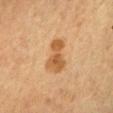No biopsy was performed on this lesion — it was imaged during a full skin examination and was not determined to be concerning. The lesion is located on the chest. The recorded lesion diameter is about 4.5 mm. A 15 mm close-up tile from a total-body photography series done for melanoma screening. Captured under cross-polarized illumination. A male subject roughly 60 years of age.